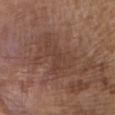location = the abdomen | patient = male, aged 73 to 77 | acquisition = 15 mm crop, total-body photography | size = ≈5.5 mm.